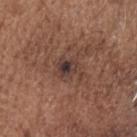Background: The patient is a male roughly 75 years of age. The tile uses white-light illumination. Located on the head or neck. About 3.5 mm across. A close-up tile cropped from a whole-body skin photograph, about 15 mm across. The lesion-visualizer software estimated a nevus-likeness score of about 35/100.